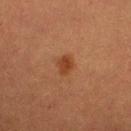Captured under cross-polarized illumination. A 15 mm crop from a total-body photograph taken for skin-cancer surveillance. A female subject, aged 53 to 57. From the left lower leg.